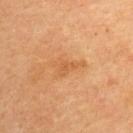Case summary:
- follow-up — imaged on a skin check; not biopsied
- imaging modality — ~15 mm tile from a whole-body skin photo
- tile lighting — cross-polarized illumination
- body site — the upper back
- automated metrics — a lesion area of about 5 mm², an eccentricity of roughly 0.85, and two-axis asymmetry of about 0.5; a border-irregularity rating of about 6/10, internal color variation of about 1 on a 0–10 scale, and a peripheral color-asymmetry measure near 0.5
- subject — female, aged 53 to 57